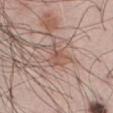<case>
<image>
  <source>total-body photography crop</source>
  <field_of_view_mm>15</field_of_view_mm>
</image>
<site>abdomen</site>
<lighting>white-light</lighting>
<lesion_size>
  <long_diameter_mm_approx>3.5</long_diameter_mm_approx>
</lesion_size>
<patient>
  <sex>male</sex>
  <age_approx>55</age_approx>
</patient>
</case>